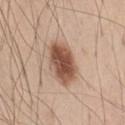Context: The lesion-visualizer software estimated a lesion area of about 6 mm², a shape eccentricity near 0.85, and two-axis asymmetry of about 0.25. The analysis additionally found a mean CIELAB color near L≈47 a*≈22 b*≈29 and a lesion–skin lightness drop of about 18. It also reported a border-irregularity rating of about 2.5/10, a within-lesion color-variation index near 2/10, and radial color variation of about 0.5. Approximately 4 mm at its widest. A male subject aged 43–47. On the chest. A region of skin cropped from a whole-body photographic capture, roughly 15 mm wide. The tile uses white-light illumination.